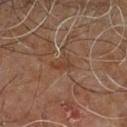biopsy_status: not biopsied; imaged during a skin examination
lesion_size:
  long_diameter_mm_approx: 3.0
lighting: cross-polarized
site: right leg
image:
  source: total-body photography crop
  field_of_view_mm: 15
patient:
  sex: male
  age_approx: 60